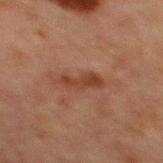Q: Was this lesion biopsied?
A: imaged on a skin check; not biopsied
Q: Illumination type?
A: cross-polarized illumination
Q: How was this image acquired?
A: total-body-photography crop, ~15 mm field of view
Q: How large is the lesion?
A: about 4.5 mm
Q: Automated lesion metrics?
A: an eccentricity of roughly 0.9 and a shape-asymmetry score of about 0.4 (0 = symmetric); a border-irregularity index near 5/10, internal color variation of about 2 on a 0–10 scale, and a peripheral color-asymmetry measure near 0.5; a classifier nevus-likeness of about 0/100 and lesion-presence confidence of about 100/100
Q: Where on the body is the lesion?
A: the chest
Q: Who is the patient?
A: male, in their mid-60s